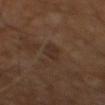Captured under cross-polarized illumination.
A male patient, in their mid- to late 60s.
The lesion's longest dimension is about 4 mm.
A 15 mm crop from a total-body photograph taken for skin-cancer surveillance.